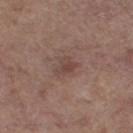* follow-up — catalogued during a skin exam; not biopsied
* image-analysis metrics — an area of roughly 4 mm², an outline eccentricity of about 0.8 (0 = round, 1 = elongated), and a symmetry-axis asymmetry near 0.4; an average lesion color of about L≈44 a*≈18 b*≈22 (CIELAB), about 8 CIELAB-L* units darker than the surrounding skin, and a normalized border contrast of about 6; a border-irregularity index near 4/10, a within-lesion color-variation index near 2.5/10, and radial color variation of about 1; a classifier nevus-likeness of about 0/100 and a detector confidence of about 100 out of 100 that the crop contains a lesion
* patient — female, aged approximately 65
* tile lighting — white-light
* location — the leg
* diameter — ~3 mm (longest diameter)
* image — 15 mm crop, total-body photography